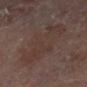biopsy status: imaged on a skin check; not biopsied | subject: female, in their 80s | site: the left leg | acquisition: 15 mm crop, total-body photography.